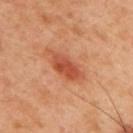biopsy status = catalogued during a skin exam; not biopsied.